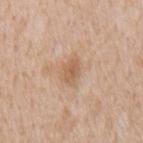Impression: Recorded during total-body skin imaging; not selected for excision or biopsy. Image and clinical context: The recorded lesion diameter is about 3.5 mm. This is a white-light tile. A male patient, roughly 65 years of age. A roughly 15 mm field-of-view crop from a total-body skin photograph. The lesion-visualizer software estimated about 9 CIELAB-L* units darker than the surrounding skin and a normalized border contrast of about 6. From the back.